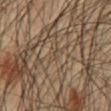Assessment: The lesion was tiled from a total-body skin photograph and was not biopsied. Context: Automated image analysis of the tile measured a lesion area of about 0.5 mm², an outline eccentricity of about 0.8 (0 = round, 1 = elongated), and a symmetry-axis asymmetry near 0.45. The analysis additionally found a mean CIELAB color near L≈44 a*≈12 b*≈25 and about 5 CIELAB-L* units darker than the surrounding skin. It also reported a border-irregularity rating of about 3.5/10 and a peripheral color-asymmetry measure near 0. On the abdomen. Cropped from a whole-body photographic skin survey; the tile spans about 15 mm. Imaged with cross-polarized lighting. A male subject aged 58 to 62.